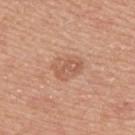This lesion was catalogued during total-body skin photography and was not selected for biopsy.
The lesion's longest dimension is about 4 mm.
Cropped from a whole-body photographic skin survey; the tile spans about 15 mm.
A male subject, aged approximately 55.
The total-body-photography lesion software estimated an eccentricity of roughly 0.7. The software also gave a classifier nevus-likeness of about 0/100 and a detector confidence of about 100 out of 100 that the crop contains a lesion.
On the upper back.